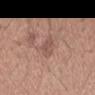<tbp_lesion>
<biopsy_status>not biopsied; imaged during a skin examination</biopsy_status>
<lighting>white-light</lighting>
<image>
  <source>total-body photography crop</source>
  <field_of_view_mm>15</field_of_view_mm>
</image>
<patient>
  <sex>male</sex>
  <age_approx>25</age_approx>
</patient>
<site>head or neck</site>
<automated_metrics>
  <cielab_L>53</cielab_L>
  <cielab_a>19</cielab_a>
  <cielab_b>24</cielab_b>
  <border_irregularity_0_10>3.5</border_irregularity_0_10>
  <color_variation_0_10>1.5</color_variation_0_10>
</automated_metrics>
</tbp_lesion>